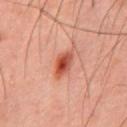Image and clinical context: An algorithmic analysis of the crop reported a detector confidence of about 100 out of 100 that the crop contains a lesion. A male patient approximately 45 years of age. Measured at roughly 3.5 mm in maximum diameter. This is a cross-polarized tile. Located on the mid back. A roughly 15 mm field-of-view crop from a total-body skin photograph.